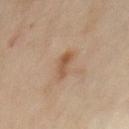{
  "biopsy_status": "not biopsied; imaged during a skin examination"
}